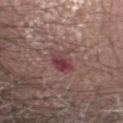<record>
<biopsy_status>not biopsied; imaged during a skin examination</biopsy_status>
<lesion_size>
  <long_diameter_mm_approx>4.5</long_diameter_mm_approx>
</lesion_size>
<patient>
  <sex>male</sex>
  <age_approx>60</age_approx>
</patient>
<site>head or neck</site>
<image>
  <source>total-body photography crop</source>
  <field_of_view_mm>15</field_of_view_mm>
</image>
</record>